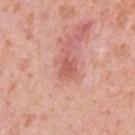Part of a total-body skin-imaging series; this lesion was reviewed on a skin check and was not flagged for biopsy. The tile uses white-light illumination. Cropped from a total-body skin-imaging series; the visible field is about 15 mm. On the arm. A female subject, aged 38–42.